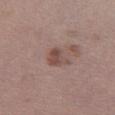Notes:
• workup — catalogued during a skin exam; not biopsied